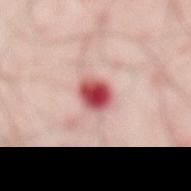{"biopsy_status": "not biopsied; imaged during a skin examination", "patient": {"sex": "male", "age_approx": 50}, "automated_metrics": {"area_mm2_approx": 6.5, "eccentricity": 0.65, "shape_asymmetry": 0.15, "cielab_L": 51, "cielab_a": 35, "cielab_b": 24, "vs_skin_darker_L": 20.0, "border_irregularity_0_10": 1.5, "color_variation_0_10": 7.5, "peripheral_color_asymmetry": 2.0}, "image": {"source": "total-body photography crop", "field_of_view_mm": 15}, "site": "abdomen", "lesion_size": {"long_diameter_mm_approx": 3.0}}